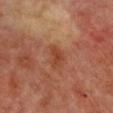No biopsy was performed on this lesion — it was imaged during a full skin examination and was not determined to be concerning.
The lesion's longest dimension is about 2.5 mm.
The subject is a female about 70 years old.
This is a cross-polarized tile.
An algorithmic analysis of the crop reported an average lesion color of about L≈37 a*≈24 b*≈30 (CIELAB), a lesion–skin lightness drop of about 7, and a lesion-to-skin contrast of about 6.5 (normalized; higher = more distinct). And it measured a border-irregularity rating of about 4.5/10, a within-lesion color-variation index near 1/10, and a peripheral color-asymmetry measure near 0.5.
On the front of the torso.
A 15 mm close-up tile from a total-body photography series done for melanoma screening.